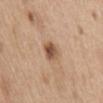  biopsy_status: not biopsied; imaged during a skin examination
  site: mid back
  patient:
    sex: male
    age_approx: 70
  image:
    source: total-body photography crop
    field_of_view_mm: 15
  lighting: white-light
  automated_metrics:
    area_mm2_approx: 5.0
    shape_asymmetry: 0.15
    cielab_L: 53
    cielab_a: 19
    cielab_b: 31
    vs_skin_darker_L: 13.0
    vs_skin_contrast_norm: 9.0